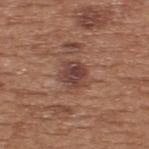Q: Is there a histopathology result?
A: no biopsy performed (imaged during a skin exam)
Q: Patient demographics?
A: male, in their mid-50s
Q: What did automated image analysis measure?
A: a lesion color around L≈42 a*≈21 b*≈24 in CIELAB, about 10 CIELAB-L* units darker than the surrounding skin, and a lesion-to-skin contrast of about 9 (normalized; higher = more distinct); a within-lesion color-variation index near 4/10; a nevus-likeness score of about 40/100
Q: Lesion size?
A: about 3 mm
Q: Where on the body is the lesion?
A: the upper back
Q: What is the imaging modality?
A: ~15 mm crop, total-body skin-cancer survey
Q: How was the tile lit?
A: white-light illumination